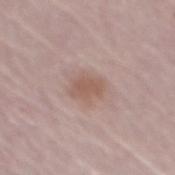<case>
<biopsy_status>not biopsied; imaged during a skin examination</biopsy_status>
<site>back</site>
<patient>
  <sex>male</sex>
  <age_approx>65</age_approx>
</patient>
<lesion_size>
  <long_diameter_mm_approx>3.0</long_diameter_mm_approx>
</lesion_size>
<lighting>white-light</lighting>
<automated_metrics>
  <area_mm2_approx>5.5</area_mm2_approx>
  <eccentricity>0.7</eccentricity>
  <border_irregularity_0_10>2.5</border_irregularity_0_10>
  <color_variation_0_10>1.5</color_variation_0_10>
  <peripheral_color_asymmetry>0.5</peripheral_color_asymmetry>
</automated_metrics>
<image>
  <source>total-body photography crop</source>
  <field_of_view_mm>15</field_of_view_mm>
</image>
</case>